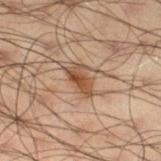biopsy status = imaged on a skin check; not biopsied | patient = male, aged around 50 | location = the right thigh | size = about 3.5 mm | image = ~15 mm tile from a whole-body skin photo.